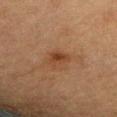| feature | finding |
|---|---|
| workup | catalogued during a skin exam; not biopsied |
| illumination | cross-polarized illumination |
| body site | the right forearm |
| diameter | about 2.5 mm |
| image | ~15 mm tile from a whole-body skin photo |
| patient | female, in their mid-50s |